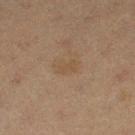{"patient": {"sex": "female", "age_approx": 20}, "site": "left thigh", "image": {"source": "total-body photography crop", "field_of_view_mm": 15}}